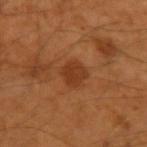Assessment: The lesion was photographed on a routine skin check and not biopsied; there is no pathology result. Background: The lesion's longest dimension is about 2.5 mm. The lesion is located on the left upper arm. Automated image analysis of the tile measured an area of roughly 6 mm², a shape eccentricity near 0.3, and a shape-asymmetry score of about 0.15 (0 = symmetric). It also reported border irregularity of about 1.5 on a 0–10 scale, a color-variation rating of about 1.5/10, and radial color variation of about 0.5. The software also gave a detector confidence of about 100 out of 100 that the crop contains a lesion. A male patient in their 50s. This is a cross-polarized tile. A lesion tile, about 15 mm wide, cut from a 3D total-body photograph.